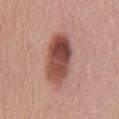Part of a total-body skin-imaging series; this lesion was reviewed on a skin check and was not flagged for biopsy. A male patient, aged around 55. The total-body-photography lesion software estimated a border-irregularity index near 2.5/10, a within-lesion color-variation index near 8.5/10, and peripheral color asymmetry of about 3. A roughly 15 mm field-of-view crop from a total-body skin photograph. The tile uses white-light illumination. Measured at roughly 7.5 mm in maximum diameter.